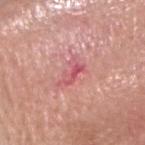| feature | finding |
|---|---|
| patient | male, aged 78 to 82 |
| location | the head or neck |
| size | about 3.5 mm |
| image | total-body-photography crop, ~15 mm field of view |
| tile lighting | white-light |